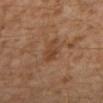Captured during whole-body skin photography for melanoma surveillance; the lesion was not biopsied. A male patient in their 30s. From the left lower leg. Measured at roughly 3 mm in maximum diameter. Cropped from a total-body skin-imaging series; the visible field is about 15 mm. Imaged with cross-polarized lighting.